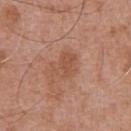biopsy_status: not biopsied; imaged during a skin examination
image:
  source: total-body photography crop
  field_of_view_mm: 15
site: chest
lighting: white-light
automated_metrics:
  cielab_L: 52
  cielab_a: 23
  cielab_b: 31
  vs_skin_darker_L: 7.0
  vs_skin_contrast_norm: 5.5
  border_irregularity_0_10: 4.5
  color_variation_0_10: 2.0
  peripheral_color_asymmetry: 0.5
  nevus_likeness_0_100: 0
  lesion_detection_confidence_0_100: 100
patient:
  sex: male
  age_approx: 75
lesion_size:
  long_diameter_mm_approx: 4.0